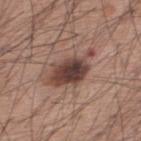workup: total-body-photography surveillance lesion; no biopsy
body site: the back
subject: male, aged around 60
imaging modality: 15 mm crop, total-body photography